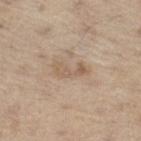Assessment:
No biopsy was performed on this lesion — it was imaged during a full skin examination and was not determined to be concerning.
Image and clinical context:
The lesion's longest dimension is about 4 mm. Imaged with white-light lighting. The patient is a male roughly 70 years of age. A close-up tile cropped from a whole-body skin photograph, about 15 mm across. The lesion-visualizer software estimated a classifier nevus-likeness of about 0/100 and a lesion-detection confidence of about 100/100. On the right thigh.